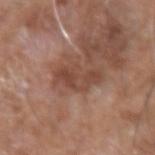Recorded during total-body skin imaging; not selected for excision or biopsy. The lesion is on the right forearm. The patient is a male aged around 75. The lesion's longest dimension is about 5 mm. Automated image analysis of the tile measured two-axis asymmetry of about 0.35. It also reported an automated nevus-likeness rating near 0 out of 100 and lesion-presence confidence of about 100/100. A lesion tile, about 15 mm wide, cut from a 3D total-body photograph.